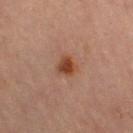  biopsy_status: not biopsied; imaged during a skin examination
  lighting: cross-polarized
  patient:
    sex: female
    age_approx: 70
  image:
    source: total-body photography crop
    field_of_view_mm: 15
  site: right thigh